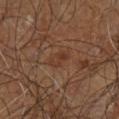Case summary:
- subject · male, roughly 60 years of age
- imaging modality · 15 mm crop, total-body photography
- TBP lesion metrics · a lesion color around L≈34 a*≈19 b*≈27 in CIELAB, roughly 5 lightness units darker than nearby skin, and a normalized border contrast of about 5; a color-variation rating of about 1/10 and radial color variation of about 0
- site · the leg
- illumination · cross-polarized
- size · ≈3 mm